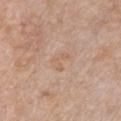This is a white-light tile.
The lesion is located on the front of the torso.
A female subject roughly 40 years of age.
A roughly 15 mm field-of-view crop from a total-body skin photograph.